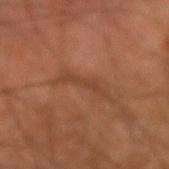{"biopsy_status": "not biopsied; imaged during a skin examination", "site": "arm", "image": {"source": "total-body photography crop", "field_of_view_mm": 15}, "patient": {"sex": "male", "age_approx": 45}, "lighting": "cross-polarized", "automated_metrics": {"border_irregularity_0_10": 8.0, "color_variation_0_10": 0.0, "peripheral_color_asymmetry": 0.0, "nevus_likeness_0_100": 0, "lesion_detection_confidence_0_100": 60}, "lesion_size": {"long_diameter_mm_approx": 5.5}}